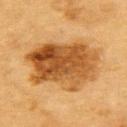<record>
<biopsy_status>not biopsied; imaged during a skin examination</biopsy_status>
<lighting>cross-polarized</lighting>
<site>upper back</site>
<image>
  <source>total-body photography crop</source>
  <field_of_view_mm>15</field_of_view_mm>
</image>
<patient>
  <sex>male</sex>
  <age_approx>85+</age_approx>
</patient>
<automated_metrics>
  <cielab_L>46</cielab_L>
  <cielab_a>22</cielab_a>
  <cielab_b>40</cielab_b>
  <vs_skin_darker_L>14.0</vs_skin_darker_L>
  <vs_skin_contrast_norm>10.5</vs_skin_contrast_norm>
  <border_irregularity_0_10>3.0</border_irregularity_0_10>
  <color_variation_0_10>8.5</color_variation_0_10>
  <peripheral_color_asymmetry>3.0</peripheral_color_asymmetry>
  <nevus_likeness_0_100>95</nevus_likeness_0_100>
  <lesion_detection_confidence_0_100>100</lesion_detection_confidence_0_100>
</automated_metrics>
</record>